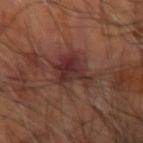No biopsy was performed on this lesion — it was imaged during a full skin examination and was not determined to be concerning. The patient is aged 63–67. A 15 mm close-up tile from a total-body photography series done for melanoma screening. From the arm. This is a cross-polarized tile. The lesion-visualizer software estimated an eccentricity of roughly 0.45 and two-axis asymmetry of about 0.3. The analysis additionally found a lesion color around L≈31 a*≈21 b*≈21 in CIELAB, about 8 CIELAB-L* units darker than the surrounding skin, and a normalized lesion–skin contrast near 8.5.